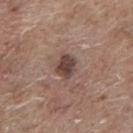| feature | finding |
|---|---|
| notes | no biopsy performed (imaged during a skin exam) |
| automated metrics | a footprint of about 6 mm² and an eccentricity of roughly 0.55; a border-irregularity rating of about 1.5/10 and a peripheral color-asymmetry measure near 1; lesion-presence confidence of about 100/100 |
| lighting | white-light |
| image source | 15 mm crop, total-body photography |
| location | the mid back |
| subject | male, aged approximately 70 |